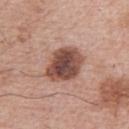Captured during whole-body skin photography for melanoma surveillance; the lesion was not biopsied.
This is a white-light tile.
The lesion is on the right upper arm.
An algorithmic analysis of the crop reported a lesion area of about 15 mm², a shape eccentricity near 0.65, and two-axis asymmetry of about 0.15. It also reported a mean CIELAB color near L≈48 a*≈22 b*≈25, roughly 17 lightness units darker than nearby skin, and a lesion-to-skin contrast of about 11.5 (normalized; higher = more distinct). The analysis additionally found border irregularity of about 1.5 on a 0–10 scale, a within-lesion color-variation index near 6/10, and a peripheral color-asymmetry measure near 1.5. And it measured a classifier nevus-likeness of about 35/100 and a detector confidence of about 100 out of 100 that the crop contains a lesion.
Longest diameter approximately 5 mm.
A region of skin cropped from a whole-body photographic capture, roughly 15 mm wide.
The subject is a male aged 63 to 67.